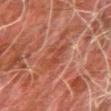| field | value |
|---|---|
| follow-up | catalogued during a skin exam; not biopsied |
| anatomic site | the arm |
| TBP lesion metrics | a footprint of about 4.5 mm², an outline eccentricity of about 0.8 (0 = round, 1 = elongated), and a symmetry-axis asymmetry near 0.35; a lesion color around L≈35 a*≈24 b*≈27 in CIELAB, about 6 CIELAB-L* units darker than the surrounding skin, and a lesion-to-skin contrast of about 6 (normalized; higher = more distinct) |
| lighting | cross-polarized |
| diameter | about 3 mm |
| image source | 15 mm crop, total-body photography |
| subject | male, approximately 80 years of age |